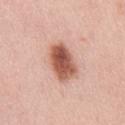Case summary:
* follow-up: catalogued during a skin exam; not biopsied
* lesion diameter: about 4.5 mm
* imaging modality: ~15 mm tile from a whole-body skin photo
* automated metrics: an area of roughly 12 mm², an eccentricity of roughly 0.8, and two-axis asymmetry of about 0.15
* anatomic site: the mid back
* subject: male, aged 48–52